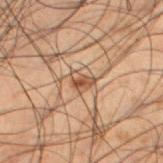Q: Was a biopsy performed?
A: total-body-photography surveillance lesion; no biopsy
Q: What is the imaging modality?
A: ~15 mm crop, total-body skin-cancer survey
Q: What is the anatomic site?
A: the left lower leg
Q: Patient demographics?
A: male, aged 48–52
Q: Automated lesion metrics?
A: a footprint of about 3.5 mm², an eccentricity of roughly 0.6, and two-axis asymmetry of about 0.45; an average lesion color of about L≈40 a*≈16 b*≈26 (CIELAB), a lesion–skin lightness drop of about 9, and a lesion-to-skin contrast of about 7.5 (normalized; higher = more distinct); radial color variation of about 1; an automated nevus-likeness rating near 55 out of 100 and a detector confidence of about 100 out of 100 that the crop contains a lesion
Q: How was the tile lit?
A: cross-polarized illumination
Q: What is the lesion's diameter?
A: ≈2.5 mm